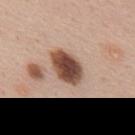Clinical impression:
The lesion was photographed on a routine skin check and not biopsied; there is no pathology result.
Acquisition and patient details:
The subject is a male aged around 45. This is a white-light tile. Cropped from a whole-body photographic skin survey; the tile spans about 15 mm. The lesion's longest dimension is about 4.5 mm. Located on the mid back.